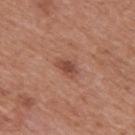Findings:
* follow-up: catalogued during a skin exam; not biopsied
* body site: the mid back
* image: ~15 mm tile from a whole-body skin photo
* patient: male, aged 68 to 72
* image-analysis metrics: a footprint of about 3.5 mm², an eccentricity of roughly 0.8, and two-axis asymmetry of about 0.25; a lesion color around L≈47 a*≈25 b*≈28 in CIELAB and a normalized border contrast of about 7.5; border irregularity of about 2.5 on a 0–10 scale and radial color variation of about 1; a detector confidence of about 100 out of 100 that the crop contains a lesion
* lesion diameter: ≈2.5 mm